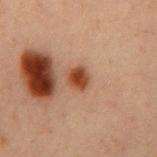notes: total-body-photography surveillance lesion; no biopsy | image source: total-body-photography crop, ~15 mm field of view | body site: the mid back | subject: male, about 60 years old | tile lighting: cross-polarized illumination | diameter: ≈2.5 mm.